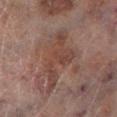biopsy status: total-body-photography surveillance lesion; no biopsy
lighting: cross-polarized
subject: male, in their mid-60s
TBP lesion metrics: a footprint of about 14 mm², a shape eccentricity near 0.9, and a shape-asymmetry score of about 0.55 (0 = symmetric); a border-irregularity index near 9/10, a color-variation rating of about 2.5/10, and radial color variation of about 0.5; a nevus-likeness score of about 0/100 and lesion-presence confidence of about 65/100
image source: ~15 mm crop, total-body skin-cancer survey
site: the left lower leg
size: ≈8 mm